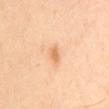follow-up = catalogued during a skin exam; not biopsied | lesion size = ~2.5 mm (longest diameter) | anatomic site = the mid back | subject = male, roughly 55 years of age | lighting = cross-polarized | imaging modality = ~15 mm crop, total-body skin-cancer survey.